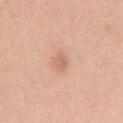body site: the front of the torso
acquisition: ~15 mm crop, total-body skin-cancer survey
illumination: white-light
patient: female, aged around 40
lesion diameter: ~3 mm (longest diameter)